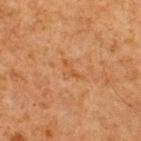Assessment: The lesion was tiled from a total-body skin photograph and was not biopsied. Image and clinical context: The lesion-visualizer software estimated an area of roughly 2.5 mm² and an outline eccentricity of about 0.85 (0 = round, 1 = elongated). It also reported a border-irregularity index near 6.5/10, a color-variation rating of about 0/10, and a peripheral color-asymmetry measure near 0. A 15 mm crop from a total-body photograph taken for skin-cancer surveillance. The subject is a male in their mid- to late 60s. The tile uses cross-polarized illumination. From the upper back. About 2.5 mm across.